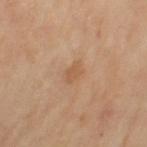Recorded during total-body skin imaging; not selected for excision or biopsy. A region of skin cropped from a whole-body photographic capture, roughly 15 mm wide. Automated image analysis of the tile measured a border-irregularity index near 2.5/10, internal color variation of about 1 on a 0–10 scale, and a peripheral color-asymmetry measure near 0.5. Measured at roughly 2.5 mm in maximum diameter. On the left thigh. The patient is a female about 70 years old. Captured under cross-polarized illumination.